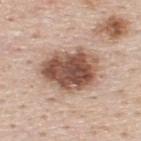Assessment:
This lesion was catalogued during total-body skin photography and was not selected for biopsy.
Acquisition and patient details:
A male patient aged 43–47. A close-up tile cropped from a whole-body skin photograph, about 15 mm across. The lesion is on the upper back.